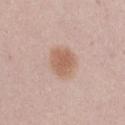Assessment:
Captured during whole-body skin photography for melanoma surveillance; the lesion was not biopsied.
Image and clinical context:
A region of skin cropped from a whole-body photographic capture, roughly 15 mm wide. A female patient, aged approximately 20. The lesion is on the right thigh. The lesion's longest dimension is about 4 mm.